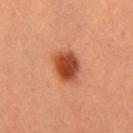The lesion was tiled from a total-body skin photograph and was not biopsied.
This is a cross-polarized tile.
A female patient, roughly 25 years of age.
A 15 mm close-up extracted from a 3D total-body photography capture.
About 4 mm across.
The total-body-photography lesion software estimated an average lesion color of about L≈46 a*≈30 b*≈36 (CIELAB) and a lesion-to-skin contrast of about 11 (normalized; higher = more distinct).
From the leg.